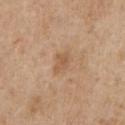Q: Is there a histopathology result?
A: total-body-photography surveillance lesion; no biopsy
Q: How large is the lesion?
A: ≈2.5 mm
Q: What is the imaging modality?
A: ~15 mm tile from a whole-body skin photo
Q: Lesion location?
A: the chest
Q: How was the tile lit?
A: white-light
Q: Who is the patient?
A: male, aged around 65
Q: Automated lesion metrics?
A: an average lesion color of about L≈56 a*≈18 b*≈34 (CIELAB) and a lesion–skin lightness drop of about 8; a border-irregularity rating of about 3/10 and radial color variation of about 0.5